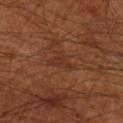No biopsy was performed on this lesion — it was imaged during a full skin examination and was not determined to be concerning. A lesion tile, about 15 mm wide, cut from a 3D total-body photograph. From the right thigh. Automated tile analysis of the lesion measured a footprint of about 5.5 mm² and an outline eccentricity of about 0.75 (0 = round, 1 = elongated). A male subject, roughly 70 years of age.